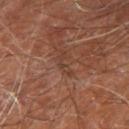{
  "image": {
    "source": "total-body photography crop",
    "field_of_view_mm": 15
  },
  "patient": {
    "sex": "male",
    "age_approx": 60
  },
  "lighting": "cross-polarized",
  "automated_metrics": {
    "cielab_L": 37,
    "cielab_a": 20,
    "cielab_b": 27,
    "vs_skin_darker_L": 6.0,
    "vs_skin_contrast_norm": 5.0
  },
  "lesion_size": {
    "long_diameter_mm_approx": 3.0
  },
  "site": "right leg"
}